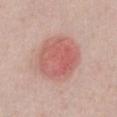Context:
Measured at roughly 6 mm in maximum diameter. Imaged with white-light lighting. A lesion tile, about 15 mm wide, cut from a 3D total-body photograph. The lesion is on the abdomen. Automated tile analysis of the lesion measured an average lesion color of about L≈59 a*≈27 b*≈26 (CIELAB) and about 10 CIELAB-L* units darker than the surrounding skin. It also reported a border-irregularity index near 1/10, a within-lesion color-variation index near 5/10, and a peripheral color-asymmetry measure near 1.5. The software also gave a nevus-likeness score of about 50/100 and lesion-presence confidence of about 100/100. A male subject, in their 40s.